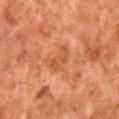The lesion was tiled from a total-body skin photograph and was not biopsied. This image is a 15 mm lesion crop taken from a total-body photograph. An algorithmic analysis of the crop reported an average lesion color of about L≈42 a*≈23 b*≈33 (CIELAB), roughly 6 lightness units darker than nearby skin, and a lesion-to-skin contrast of about 5.5 (normalized; higher = more distinct). The lesion is on the right lower leg. Imaged with cross-polarized lighting. The patient is a male approximately 80 years of age.